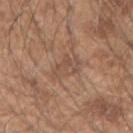The lesion was photographed on a routine skin check and not biopsied; there is no pathology result. Automated image analysis of the tile measured a lesion color around L≈50 a*≈18 b*≈28 in CIELAB and a lesion-to-skin contrast of about 5 (normalized; higher = more distinct). And it measured an automated nevus-likeness rating near 0 out of 100. A 15 mm crop from a total-body photograph taken for skin-cancer surveillance. The lesion is on the right forearm. A male subject aged approximately 50. Imaged with white-light lighting. Measured at roughly 3.5 mm in maximum diameter.